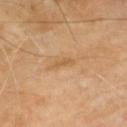The lesion was tiled from a total-body skin photograph and was not biopsied.
A male patient aged 68–72.
This image is a 15 mm lesion crop taken from a total-body photograph.
The tile uses cross-polarized illumination.
The lesion is located on the chest.